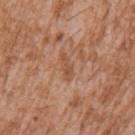Captured during whole-body skin photography for melanoma surveillance; the lesion was not biopsied.
A male subject aged 43–47.
The recorded lesion diameter is about 3 mm.
Captured under white-light illumination.
The total-body-photography lesion software estimated a nevus-likeness score of about 0/100 and a detector confidence of about 100 out of 100 that the crop contains a lesion.
This image is a 15 mm lesion crop taken from a total-body photograph.
The lesion is located on the left upper arm.